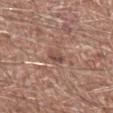workup: imaged on a skin check; not biopsied | body site: the right lower leg | subject: male, about 80 years old | acquisition: 15 mm crop, total-body photography | lesion size: about 2.5 mm.